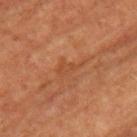Q: Is there a histopathology result?
A: no biopsy performed (imaged during a skin exam)
Q: How large is the lesion?
A: ≈3.5 mm
Q: Automated lesion metrics?
A: a footprint of about 4 mm² and an eccentricity of roughly 0.85; an average lesion color of about L≈44 a*≈24 b*≈34 (CIELAB), about 5 CIELAB-L* units darker than the surrounding skin, and a lesion-to-skin contrast of about 4.5 (normalized; higher = more distinct); a border-irregularity index near 6/10, internal color variation of about 1 on a 0–10 scale, and radial color variation of about 0.5; a classifier nevus-likeness of about 0/100 and a detector confidence of about 100 out of 100 that the crop contains a lesion
Q: What is the anatomic site?
A: the chest
Q: Illumination type?
A: cross-polarized illumination
Q: What is the imaging modality?
A: total-body-photography crop, ~15 mm field of view
Q: Who is the patient?
A: male, aged 63 to 67